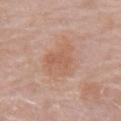<tbp_lesion>
  <biopsy_status>not biopsied; imaged during a skin examination</biopsy_status>
  <lesion_size>
    <long_diameter_mm_approx>5.0</long_diameter_mm_approx>
  </lesion_size>
  <lighting>white-light</lighting>
  <image>
    <source>total-body photography crop</source>
    <field_of_view_mm>15</field_of_view_mm>
  </image>
  <automated_metrics>
    <area_mm2_approx>14.0</area_mm2_approx>
    <shape_asymmetry>0.25</shape_asymmetry>
    <cielab_L>60</cielab_L>
    <cielab_a>21</cielab_a>
    <cielab_b>30</cielab_b>
    <vs_skin_contrast_norm>5.5</vs_skin_contrast_norm>
    <nevus_likeness_0_100>5</nevus_likeness_0_100>
    <lesion_detection_confidence_0_100>100</lesion_detection_confidence_0_100>
  </automated_metrics>
  <patient>
    <sex>female</sex>
    <age_approx>65</age_approx>
  </patient>
  <site>right upper arm</site>
</tbp_lesion>